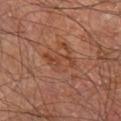This lesion was catalogued during total-body skin photography and was not selected for biopsy. A lesion tile, about 15 mm wide, cut from a 3D total-body photograph. On the leg. The recorded lesion diameter is about 4 mm. A male subject aged around 60.